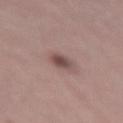Impression: Part of a total-body skin-imaging series; this lesion was reviewed on a skin check and was not flagged for biopsy. Image and clinical context: Approximately 3 mm at its widest. A region of skin cropped from a whole-body photographic capture, roughly 15 mm wide. The subject is a female aged 43 to 47. Imaged with white-light lighting. Located on the right thigh. Automated tile analysis of the lesion measured a lesion–skin lightness drop of about 12 and a normalized lesion–skin contrast near 8.5.